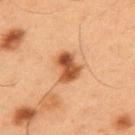Recorded during total-body skin imaging; not selected for excision or biopsy.
Cropped from a total-body skin-imaging series; the visible field is about 15 mm.
A male subject approximately 55 years of age.
From the upper back.